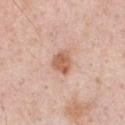Captured under white-light illumination. The subject is a male approximately 50 years of age. Measured at roughly 3 mm in maximum diameter. Cropped from a total-body skin-imaging series; the visible field is about 15 mm. On the chest.